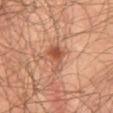Case summary:
– biopsy status · imaged on a skin check; not biopsied
– image-analysis metrics · an area of roughly 6 mm² and an eccentricity of roughly 0.65; border irregularity of about 5 on a 0–10 scale and a color-variation rating of about 4.5/10; an automated nevus-likeness rating near 85 out of 100 and a detector confidence of about 100 out of 100 that the crop contains a lesion
– acquisition · total-body-photography crop, ~15 mm field of view
– location · the lower back
– patient · male, aged approximately 45